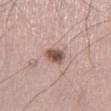notes=no biopsy performed (imaged during a skin exam) | patient=male, approximately 45 years of age | site=the left thigh | image source=total-body-photography crop, ~15 mm field of view.